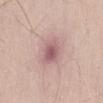The lesion was tiled from a total-body skin photograph and was not biopsied.
The lesion's longest dimension is about 4 mm.
The lesion is on the front of the torso.
Automated tile analysis of the lesion measured an average lesion color of about L≈59 a*≈22 b*≈19 (CIELAB), a lesion–skin lightness drop of about 11, and a lesion-to-skin contrast of about 7 (normalized; higher = more distinct). The software also gave a border-irregularity rating of about 2/10 and internal color variation of about 4.5 on a 0–10 scale. It also reported a classifier nevus-likeness of about 0/100 and a detector confidence of about 100 out of 100 that the crop contains a lesion.
A 15 mm crop from a total-body photograph taken for skin-cancer surveillance.
Imaged with white-light lighting.
A male subject, aged 48–52.